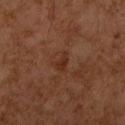Clinical impression:
Captured during whole-body skin photography for melanoma surveillance; the lesion was not biopsied.
Acquisition and patient details:
The patient is a female aged 58–62. Located on the right upper arm. A 15 mm close-up extracted from a 3D total-body photography capture.